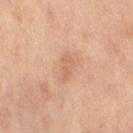Q: Was a biopsy performed?
A: imaged on a skin check; not biopsied
Q: What lighting was used for the tile?
A: cross-polarized illumination
Q: Where on the body is the lesion?
A: the right thigh
Q: Lesion size?
A: ~2.5 mm (longest diameter)
Q: Who is the patient?
A: female, aged approximately 60
Q: What kind of image is this?
A: total-body-photography crop, ~15 mm field of view